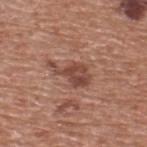This lesion was catalogued during total-body skin photography and was not selected for biopsy.
From the upper back.
A male subject in their mid- to late 70s.
Captured under white-light illumination.
Longest diameter approximately 4.5 mm.
A roughly 15 mm field-of-view crop from a total-body skin photograph.